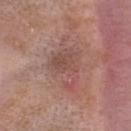notes = catalogued during a skin exam; not biopsied | lesion size = ~6.5 mm (longest diameter) | tile lighting = white-light illumination | subject = female, aged approximately 25 | anatomic site = the head or neck | image source = ~15 mm tile from a whole-body skin photo.